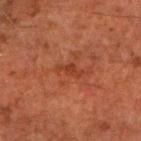Part of a total-body skin-imaging series; this lesion was reviewed on a skin check and was not flagged for biopsy. A male patient in their 60s. From the right forearm. Automated image analysis of the tile measured an area of roughly 2.5 mm², an outline eccentricity of about 0.9 (0 = round, 1 = elongated), and two-axis asymmetry of about 0.4. Cropped from a whole-body photographic skin survey; the tile spans about 15 mm. Approximately 3 mm at its widest.